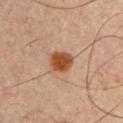notes: total-body-photography surveillance lesion; no biopsy
TBP lesion metrics: a lesion area of about 6.5 mm² and an eccentricity of roughly 0.5
imaging modality: ~15 mm crop, total-body skin-cancer survey
illumination: cross-polarized illumination
subject: male, aged approximately 60
lesion size: ≈3 mm
body site: the chest